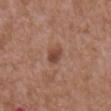| field | value |
|---|---|
| biopsy status | catalogued during a skin exam; not biopsied |
| anatomic site | the abdomen |
| tile lighting | white-light |
| patient | male, roughly 75 years of age |
| image source | total-body-photography crop, ~15 mm field of view |